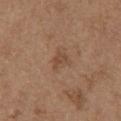| field | value |
|---|---|
| patient | female, aged approximately 65 |
| location | the chest |
| imaging modality | ~15 mm tile from a whole-body skin photo |
| tile lighting | white-light illumination |